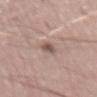Impression:
The lesion was photographed on a routine skin check and not biopsied; there is no pathology result.
Background:
Approximately 3 mm at its widest. A region of skin cropped from a whole-body photographic capture, roughly 15 mm wide. A male subject, aged around 55. The lesion is located on the abdomen. Automated image analysis of the tile measured a lesion color around L≈53 a*≈17 b*≈22 in CIELAB, roughly 11 lightness units darker than nearby skin, and a normalized lesion–skin contrast near 7.5. It also reported a within-lesion color-variation index near 2.5/10. And it measured an automated nevus-likeness rating near 0 out of 100 and lesion-presence confidence of about 95/100.